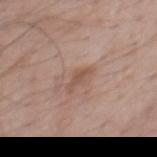Recorded during total-body skin imaging; not selected for excision or biopsy.
The lesion is located on the front of the torso.
Measured at roughly 3.5 mm in maximum diameter.
The tile uses white-light illumination.
A male patient, aged 68–72.
Automated tile analysis of the lesion measured a nevus-likeness score of about 0/100.
A close-up tile cropped from a whole-body skin photograph, about 15 mm across.